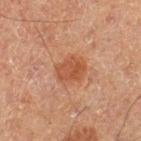Q: Is there a histopathology result?
A: no biopsy performed (imaged during a skin exam)
Q: Lesion location?
A: the leg
Q: What kind of image is this?
A: ~15 mm tile from a whole-body skin photo
Q: What are the patient's age and sex?
A: male, about 65 years old
Q: How was the tile lit?
A: cross-polarized